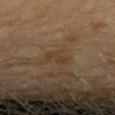Assessment: The lesion was tiled from a total-body skin photograph and was not biopsied. Background: From the right forearm. A roughly 15 mm field-of-view crop from a total-body skin photograph. Measured at roughly 2.5 mm in maximum diameter. The subject is a female aged approximately 60. This is a cross-polarized tile.